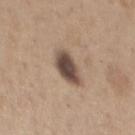Clinical impression: No biopsy was performed on this lesion — it was imaged during a full skin examination and was not determined to be concerning. Clinical summary: A lesion tile, about 15 mm wide, cut from a 3D total-body photograph. A male patient, roughly 65 years of age. The lesion's longest dimension is about 4 mm. The lesion is located on the chest.